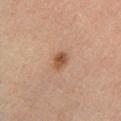Findings:
– notes — no biopsy performed (imaged during a skin exam)
– illumination — cross-polarized illumination
– site — the leg
– imaging modality — total-body-photography crop, ~15 mm field of view
– subject — female, approximately 55 years of age
– diameter — ≈2.5 mm